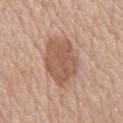follow-up = imaged on a skin check; not biopsied
subject = male, about 75 years old
diameter = about 6.5 mm
anatomic site = the chest
illumination = white-light
automated lesion analysis = a color-variation rating of about 2.5/10 and a peripheral color-asymmetry measure near 1
image = ~15 mm crop, total-body skin-cancer survey